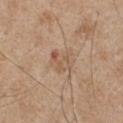The lesion was tiled from a total-body skin photograph and was not biopsied.
The patient is a male about 65 years old.
The tile uses white-light illumination.
From the chest.
Approximately 3 mm at its widest.
A close-up tile cropped from a whole-body skin photograph, about 15 mm across.